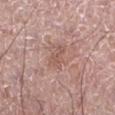Q: Was a biopsy performed?
A: imaged on a skin check; not biopsied
Q: How was this image acquired?
A: total-body-photography crop, ~15 mm field of view
Q: Patient demographics?
A: male, aged 58 to 62
Q: Lesion size?
A: about 3 mm
Q: What lighting was used for the tile?
A: white-light
Q: What is the anatomic site?
A: the left lower leg